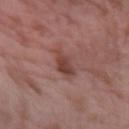follow-up: catalogued during a skin exam; not biopsied
tile lighting: white-light illumination
subject: male, aged 38–42
size: about 3 mm
automated lesion analysis: a shape eccentricity near 0.6 and a shape-asymmetry score of about 0.25 (0 = symmetric); a mean CIELAB color near L≈43 a*≈23 b*≈24, about 10 CIELAB-L* units darker than the surrounding skin, and a normalized border contrast of about 8; a nevus-likeness score of about 25/100 and a lesion-detection confidence of about 100/100
image source: 15 mm crop, total-body photography
body site: the left forearm